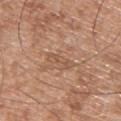Approximately 3.5 mm at its widest. This image is a 15 mm lesion crop taken from a total-body photograph. The lesion is on the upper back. A male patient about 50 years old.